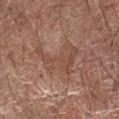The lesion was tiled from a total-body skin photograph and was not biopsied.
Automated image analysis of the tile measured an eccentricity of roughly 0.75. It also reported a lesion–skin lightness drop of about 6 and a normalized border contrast of about 5. And it measured a border-irregularity rating of about 8/10, a within-lesion color-variation index near 3/10, and peripheral color asymmetry of about 1. It also reported a classifier nevus-likeness of about 0/100 and a lesion-detection confidence of about 85/100.
About 5.5 mm across.
The lesion is located on the left forearm.
A close-up tile cropped from a whole-body skin photograph, about 15 mm across.
Captured under white-light illumination.
The subject is a female roughly 80 years of age.